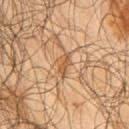follow-up: imaged on a skin check; not biopsied | anatomic site: the right upper arm | size: ~3 mm (longest diameter) | acquisition: 15 mm crop, total-body photography | illumination: cross-polarized | subject: male, aged 63–67.